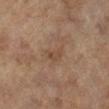Impression:
Part of a total-body skin-imaging series; this lesion was reviewed on a skin check and was not flagged for biopsy.
Clinical summary:
A female subject aged around 60. The lesion is located on the left lower leg. The recorded lesion diameter is about 2.5 mm. This is a cross-polarized tile. This image is a 15 mm lesion crop taken from a total-body photograph.